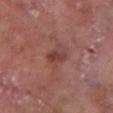Part of a total-body skin-imaging series; this lesion was reviewed on a skin check and was not flagged for biopsy.